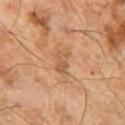Q: What are the patient's age and sex?
A: male, approximately 65 years of age
Q: Automated lesion metrics?
A: a footprint of about 3.5 mm² and a symmetry-axis asymmetry near 0.45; an average lesion color of about L≈54 a*≈21 b*≈35 (CIELAB) and about 8 CIELAB-L* units darker than the surrounding skin
Q: How large is the lesion?
A: ~2.5 mm (longest diameter)
Q: What kind of image is this?
A: 15 mm crop, total-body photography